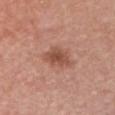{"biopsy_status": "not biopsied; imaged during a skin examination", "site": "chest", "patient": {"sex": "female", "age_approx": 60}, "image": {"source": "total-body photography crop", "field_of_view_mm": 15}}